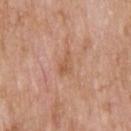Located on the upper back. An algorithmic analysis of the crop reported an outline eccentricity of about 0.85 (0 = round, 1 = elongated) and a symmetry-axis asymmetry near 0.4. It also reported a nevus-likeness score of about 0/100. This image is a 15 mm lesion crop taken from a total-body photograph. A male subject about 60 years old.